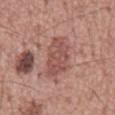* anatomic site: the mid back
* tile lighting: white-light illumination
* patient: male, in their mid- to late 50s
* imaging modality: 15 mm crop, total-body photography
* diameter: ≈5.5 mm